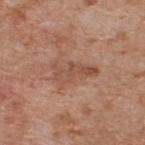<record>
<lighting>white-light</lighting>
<lesion_size>
  <long_diameter_mm_approx>5.0</long_diameter_mm_approx>
</lesion_size>
<patient>
  <sex>male</sex>
  <age_approx>60</age_approx>
</patient>
<automated_metrics>
  <area_mm2_approx>9.5</area_mm2_approx>
  <eccentricity>0.9</eccentricity>
  <shape_asymmetry>0.45</shape_asymmetry>
  <cielab_L>51</cielab_L>
  <cielab_a>21</cielab_a>
  <cielab_b>29</cielab_b>
  <vs_skin_contrast_norm>5.5</vs_skin_contrast_norm>
</automated_metrics>
<site>upper back</site>
<image>
  <source>total-body photography crop</source>
  <field_of_view_mm>15</field_of_view_mm>
</image>
</record>